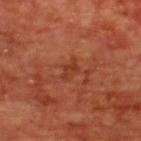notes = imaged on a skin check; not biopsied | location = the upper back | subject = male, about 65 years old | image source = total-body-photography crop, ~15 mm field of view.